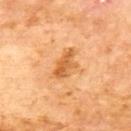No biopsy was performed on this lesion — it was imaged during a full skin examination and was not determined to be concerning.
Cropped from a total-body skin-imaging series; the visible field is about 15 mm.
A male patient about 70 years old.
On the upper back.